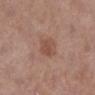Part of a total-body skin-imaging series; this lesion was reviewed on a skin check and was not flagged for biopsy. The patient is a female aged 58–62. A close-up tile cropped from a whole-body skin photograph, about 15 mm across. Imaged with white-light lighting. The recorded lesion diameter is about 3 mm. From the right lower leg.